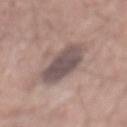follow-up = imaged on a skin check; not biopsied.